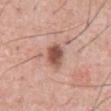| feature | finding |
|---|---|
| notes | no biopsy performed (imaged during a skin exam) |
| patient | male, aged 58 to 62 |
| diameter | about 3.5 mm |
| lighting | white-light |
| site | the abdomen |
| image | ~15 mm crop, total-body skin-cancer survey |